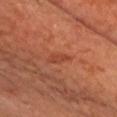Impression: Imaged during a routine full-body skin examination; the lesion was not biopsied and no histopathology is available. Background: This is a cross-polarized tile. Approximately 3 mm at its widest. A 15 mm close-up tile from a total-body photography series done for melanoma screening. A male subject aged 63 to 67. Located on the head or neck. An algorithmic analysis of the crop reported a mean CIELAB color near L≈43 a*≈28 b*≈34, roughly 6 lightness units darker than nearby skin, and a normalized lesion–skin contrast near 5. It also reported a border-irregularity rating of about 3.5/10 and radial color variation of about 0. It also reported an automated nevus-likeness rating near 5 out of 100.